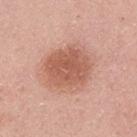Notes:
- follow-up — no biopsy performed (imaged during a skin exam)
- lesion diameter — ~5.5 mm (longest diameter)
- illumination — white-light
- location — the upper back
- TBP lesion metrics — border irregularity of about 1.5 on a 0–10 scale and internal color variation of about 3 on a 0–10 scale
- patient — male, aged 38–42
- image — ~15 mm tile from a whole-body skin photo